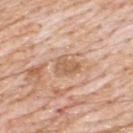  biopsy_status: not biopsied; imaged during a skin examination
  site: back
  lesion_size:
    long_diameter_mm_approx: 3.0
  patient:
    sex: male
    age_approx: 80
  image:
    source: total-body photography crop
    field_of_view_mm: 15
  lighting: white-light